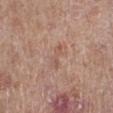{
  "biopsy_status": "not biopsied; imaged during a skin examination",
  "patient": {
    "sex": "male",
    "age_approx": 80
  },
  "image": {
    "source": "total-body photography crop",
    "field_of_view_mm": 15
  },
  "automated_metrics": {
    "area_mm2_approx": 3.0,
    "eccentricity": 0.95,
    "shape_asymmetry": 0.3,
    "cielab_L": 55,
    "cielab_a": 20,
    "cielab_b": 27,
    "vs_skin_contrast_norm": 4.5,
    "border_irregularity_0_10": 4.5,
    "color_variation_0_10": 0.0,
    "peripheral_color_asymmetry": 0.0
  },
  "lighting": "white-light",
  "site": "left leg",
  "lesion_size": {
    "long_diameter_mm_approx": 3.0
  }
}